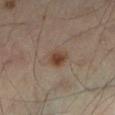site: left lower leg
image:
  source: total-body photography crop
  field_of_view_mm: 15
patient:
  sex: male
  age_approx: 55